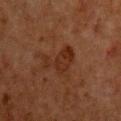workup — catalogued during a skin exam; not biopsied | location — the chest | size — ≈5 mm | acquisition — ~15 mm crop, total-body skin-cancer survey | illumination — cross-polarized illumination | patient — male, in their mid-60s | image-analysis metrics — a footprint of about 9.5 mm², an outline eccentricity of about 0.85 (0 = round, 1 = elongated), and a symmetry-axis asymmetry near 0.55; a lesion color around L≈25 a*≈20 b*≈27 in CIELAB, a lesion–skin lightness drop of about 6, and a normalized border contrast of about 6.5; lesion-presence confidence of about 100/100.